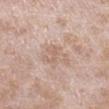workup: no biopsy performed (imaged during a skin exam)
patient: female, in their 40s
size: about 2.5 mm
lighting: white-light illumination
body site: the leg
image: ~15 mm tile from a whole-body skin photo